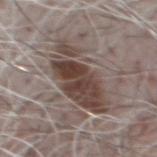notes: total-body-photography surveillance lesion; no biopsy | lighting: white-light illumination | body site: the chest | automated metrics: a lesion area of about 23 mm², a shape eccentricity near 0.85, and a shape-asymmetry score of about 0.3 (0 = symmetric); an average lesion color of about L≈42 a*≈14 b*≈20 (CIELAB), roughly 13 lightness units darker than nearby skin, and a lesion-to-skin contrast of about 10.5 (normalized; higher = more distinct); an automated nevus-likeness rating near 95 out of 100 and a lesion-detection confidence of about 60/100 | subject: male, in their 70s | size: about 7.5 mm | image source: ~15 mm tile from a whole-body skin photo.